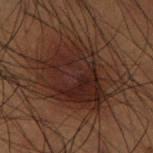Captured during whole-body skin photography for melanoma surveillance; the lesion was not biopsied.
The lesion is located on the right lower leg.
Cropped from a whole-body photographic skin survey; the tile spans about 15 mm.
Captured under cross-polarized illumination.
Automated image analysis of the tile measured an area of roughly 40 mm², an eccentricity of roughly 0.65, and a symmetry-axis asymmetry near 0.2.
The patient is a male aged approximately 50.
The lesion's longest dimension is about 7.5 mm.